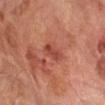– notes — imaged on a skin check; not biopsied
– lesion diameter — about 3 mm
– image — ~15 mm tile from a whole-body skin photo
– subject — male, in their 70s
– site — the arm
– tile lighting — cross-polarized illumination
– automated metrics — a footprint of about 4 mm²; a lesion color around L≈45 a*≈30 b*≈30 in CIELAB and a lesion–skin lightness drop of about 9; a border-irregularity index near 2.5/10, a color-variation rating of about 2.5/10, and a peripheral color-asymmetry measure near 1